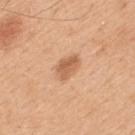Imaged during a routine full-body skin examination; the lesion was not biopsied and no histopathology is available.
The subject is a male about 50 years old.
Cropped from a total-body skin-imaging series; the visible field is about 15 mm.
The tile uses white-light illumination.
The lesion is on the mid back.
The lesion's longest dimension is about 3 mm.